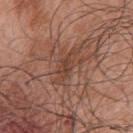Case summary:
• notes: imaged on a skin check; not biopsied
• diameter: ≈4.5 mm
• imaging modality: 15 mm crop, total-body photography
• lighting: white-light
• site: the chest
• image-analysis metrics: a lesion color around L≈43 a*≈21 b*≈27 in CIELAB and roughly 7 lightness units darker than nearby skin; a border-irregularity rating of about 5/10, a within-lesion color-variation index near 2/10, and peripheral color asymmetry of about 0.5; a classifier nevus-likeness of about 0/100 and a detector confidence of about 90 out of 100 that the crop contains a lesion
• patient: male, in their 70s